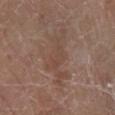Imaged during a routine full-body skin examination; the lesion was not biopsied and no histopathology is available. Longest diameter approximately 7 mm. The subject is a female aged around 80. From the right lower leg. A close-up tile cropped from a whole-body skin photograph, about 15 mm across. Automated image analysis of the tile measured a border-irregularity index near 9/10 and a peripheral color-asymmetry measure near 1. It also reported lesion-presence confidence of about 95/100. Imaged with white-light lighting.